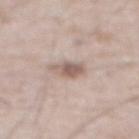Clinical impression: No biopsy was performed on this lesion — it was imaged during a full skin examination and was not determined to be concerning. Clinical summary: A close-up tile cropped from a whole-body skin photograph, about 15 mm across. Longest diameter approximately 3 mm. The lesion-visualizer software estimated a lesion area of about 5.5 mm², an outline eccentricity of about 0.7 (0 = round, 1 = elongated), and a shape-asymmetry score of about 0.2 (0 = symmetric). It also reported a mean CIELAB color near L≈59 a*≈16 b*≈23, about 11 CIELAB-L* units darker than the surrounding skin, and a normalized border contrast of about 7.5. The software also gave a classifier nevus-likeness of about 60/100. Located on the front of the torso. A male patient, about 65 years old. Captured under white-light illumination.